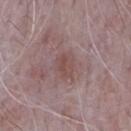Imaged during a routine full-body skin examination; the lesion was not biopsied and no histopathology is available.
About 3 mm across.
A male patient, in their mid- to late 60s.
A 15 mm crop from a total-body photograph taken for skin-cancer surveillance.
The lesion is located on the front of the torso.
The tile uses white-light illumination.